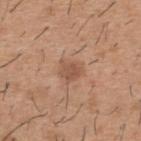Impression:
The lesion was photographed on a routine skin check and not biopsied; there is no pathology result.
Image and clinical context:
The tile uses white-light illumination. A region of skin cropped from a whole-body photographic capture, roughly 15 mm wide. The lesion-visualizer software estimated a classifier nevus-likeness of about 0/100 and lesion-presence confidence of about 100/100. The subject is a male in their 40s. Longest diameter approximately 2.5 mm. From the back.